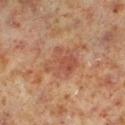Impression:
The lesion was tiled from a total-body skin photograph and was not biopsied.
Image and clinical context:
The tile uses cross-polarized illumination. A male subject in their 60s. The lesion is on the left lower leg. This image is a 15 mm lesion crop taken from a total-body photograph.